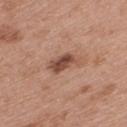biopsy_status: not biopsied; imaged during a skin examination
site: upper back
image:
  source: total-body photography crop
  field_of_view_mm: 15
patient:
  sex: female
  age_approx: 40